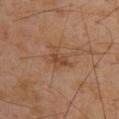Imaged during a routine full-body skin examination; the lesion was not biopsied and no histopathology is available. This image is a 15 mm lesion crop taken from a total-body photograph. A male subject, approximately 50 years of age. Measured at roughly 3.5 mm in maximum diameter. An algorithmic analysis of the crop reported a shape eccentricity near 0.65. The analysis additionally found a mean CIELAB color near L≈45 a*≈20 b*≈32, roughly 7 lightness units darker than nearby skin, and a normalized lesion–skin contrast near 6. It also reported a border-irregularity index near 5/10, a color-variation rating of about 2.5/10, and a peripheral color-asymmetry measure near 1. From the upper back. The tile uses cross-polarized illumination.